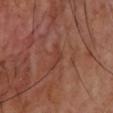Impression: This lesion was catalogued during total-body skin photography and was not selected for biopsy. Clinical summary: On the upper back. A male subject, about 65 years old. The lesion-visualizer software estimated a mean CIELAB color near L≈39 a*≈24 b*≈28. It also reported a nevus-likeness score of about 0/100. A close-up tile cropped from a whole-body skin photograph, about 15 mm across.